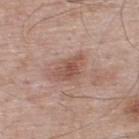Q: Is there a histopathology result?
A: no biopsy performed (imaged during a skin exam)
Q: Illumination type?
A: white-light
Q: Where on the body is the lesion?
A: the back
Q: How was this image acquired?
A: ~15 mm tile from a whole-body skin photo
Q: Automated lesion metrics?
A: a lesion area of about 7 mm², a shape eccentricity near 0.8, and a symmetry-axis asymmetry near 0.3; a nevus-likeness score of about 5/100
Q: How large is the lesion?
A: about 4 mm
Q: What are the patient's age and sex?
A: male, aged approximately 65